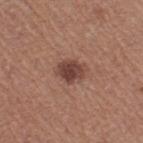This lesion was catalogued during total-body skin photography and was not selected for biopsy.
Captured under white-light illumination.
A lesion tile, about 15 mm wide, cut from a 3D total-body photograph.
Measured at roughly 3.5 mm in maximum diameter.
A female subject, roughly 50 years of age.
Located on the right thigh.